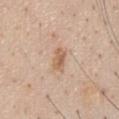Recorded during total-body skin imaging; not selected for excision or biopsy. This image is a 15 mm lesion crop taken from a total-body photograph. On the chest. Approximately 3 mm at its widest. A male subject, roughly 60 years of age. Captured under white-light illumination.